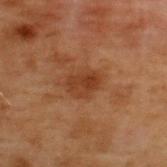Case summary:
– patient — male, roughly 70 years of age
– anatomic site — the upper back
– imaging modality — 15 mm crop, total-body photography